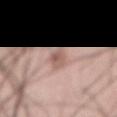Captured during whole-body skin photography for melanoma surveillance; the lesion was not biopsied.
The tile uses white-light illumination.
The lesion is on the abdomen.
The subject is a male aged around 60.
Approximately 4 mm at its widest.
A roughly 15 mm field-of-view crop from a total-body skin photograph.